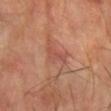This lesion was catalogued during total-body skin photography and was not selected for biopsy. The patient is a male aged approximately 70. A roughly 15 mm field-of-view crop from a total-body skin photograph. This is a cross-polarized tile. Located on the left forearm. The lesion's longest dimension is about 2.5 mm.